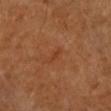The lesion was tiled from a total-body skin photograph and was not biopsied. The recorded lesion diameter is about 2.5 mm. From the left arm. The tile uses cross-polarized illumination. The subject is a female aged 58–62. Automated image analysis of the tile measured a lesion area of about 2 mm², an outline eccentricity of about 0.95 (0 = round, 1 = elongated), and two-axis asymmetry of about 0.3. The analysis additionally found a mean CIELAB color near L≈41 a*≈26 b*≈36, a lesion–skin lightness drop of about 5, and a normalized lesion–skin contrast near 5. It also reported a border-irregularity rating of about 3.5/10, internal color variation of about 0 on a 0–10 scale, and a peripheral color-asymmetry measure near 0. A roughly 15 mm field-of-view crop from a total-body skin photograph.